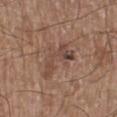follow-up = catalogued during a skin exam; not biopsied